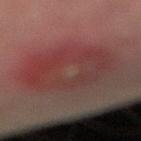The lesion was photographed on a routine skin check and not biopsied; there is no pathology result.
On the right lower leg.
A roughly 15 mm field-of-view crop from a total-body skin photograph.
The patient is a male in their mid-70s.
This is a cross-polarized tile.
Approximately 8 mm at its widest.